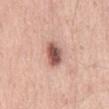The lesion was tiled from a total-body skin photograph and was not biopsied. A male subject, in their mid-50s. A lesion tile, about 15 mm wide, cut from a 3D total-body photograph. The lesion-visualizer software estimated an area of roughly 7 mm², an eccentricity of roughly 0.8, and a shape-asymmetry score of about 0.25 (0 = symmetric). It also reported an average lesion color of about L≈56 a*≈22 b*≈26 (CIELAB), about 17 CIELAB-L* units darker than the surrounding skin, and a lesion-to-skin contrast of about 10.5 (normalized; higher = more distinct). The analysis additionally found a detector confidence of about 100 out of 100 that the crop contains a lesion. From the abdomen. The lesion's longest dimension is about 4 mm. The tile uses white-light illumination.